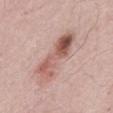Assessment: No biopsy was performed on this lesion — it was imaged during a full skin examination and was not determined to be concerning. Image and clinical context: Captured under white-light illumination. The recorded lesion diameter is about 7 mm. An algorithmic analysis of the crop reported a footprint of about 15 mm², a shape eccentricity near 0.95, and two-axis asymmetry of about 0.25. And it measured a mean CIELAB color near L≈57 a*≈22 b*≈25, about 13 CIELAB-L* units darker than the surrounding skin, and a normalized border contrast of about 8.5. It also reported a border-irregularity rating of about 4/10, a within-lesion color-variation index near 9.5/10, and peripheral color asymmetry of about 4.5. The analysis additionally found a nevus-likeness score of about 70/100 and a detector confidence of about 100 out of 100 that the crop contains a lesion. From the mid back. This image is a 15 mm lesion crop taken from a total-body photograph. A male subject, aged 63–67.